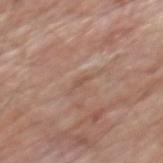Clinical impression:
Imaged during a routine full-body skin examination; the lesion was not biopsied and no histopathology is available.
Image and clinical context:
The total-body-photography lesion software estimated a mean CIELAB color near L≈52 a*≈20 b*≈28 and a lesion–skin lightness drop of about 7. Located on the mid back. Approximately 1.5 mm at its widest. A 15 mm crop from a total-body photograph taken for skin-cancer surveillance. A male patient, about 80 years old. The tile uses white-light illumination.